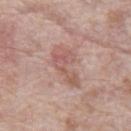follow-up: catalogued during a skin exam; not biopsied | illumination: white-light | automated lesion analysis: a shape eccentricity near 0.85 and a symmetry-axis asymmetry near 0.4 | lesion diameter: ≈5 mm | site: the abdomen | imaging modality: ~15 mm crop, total-body skin-cancer survey | patient: male, aged 68 to 72.